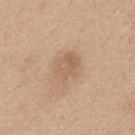Q: Was this lesion biopsied?
A: no biopsy performed (imaged during a skin exam)
Q: Lesion size?
A: ≈4 mm
Q: Lesion location?
A: the back
Q: What kind of image is this?
A: 15 mm crop, total-body photography
Q: Patient demographics?
A: male, aged 58–62
Q: How was the tile lit?
A: white-light illumination